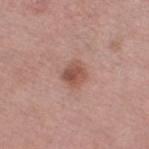No biopsy was performed on this lesion — it was imaged during a full skin examination and was not determined to be concerning. Longest diameter approximately 2.5 mm. A female subject, aged around 70. A roughly 15 mm field-of-view crop from a total-body skin photograph. The lesion is on the leg. Captured under white-light illumination.